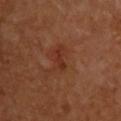{"biopsy_status": "not biopsied; imaged during a skin examination", "lesion_size": {"long_diameter_mm_approx": 2.5}, "image": {"source": "total-body photography crop", "field_of_view_mm": 15}, "lighting": "cross-polarized", "patient": {"sex": "female", "age_approx": 65}, "site": "upper back"}